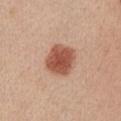The lesion was photographed on a routine skin check and not biopsied; there is no pathology result.
A region of skin cropped from a whole-body photographic capture, roughly 15 mm wide.
Automated tile analysis of the lesion measured a footprint of about 12 mm², an eccentricity of roughly 0.45, and a symmetry-axis asymmetry near 0.1. The analysis additionally found an average lesion color of about L≈53 a*≈25 b*≈31 (CIELAB) and about 15 CIELAB-L* units darker than the surrounding skin. And it measured border irregularity of about 1 on a 0–10 scale, internal color variation of about 3 on a 0–10 scale, and radial color variation of about 1. And it measured a classifier nevus-likeness of about 100/100 and a detector confidence of about 100 out of 100 that the crop contains a lesion.
The lesion is located on the arm.
Captured under white-light illumination.
Longest diameter approximately 4 mm.
The patient is a male about 75 years old.